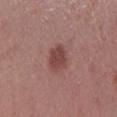This lesion was catalogued during total-body skin photography and was not selected for biopsy. The lesion's longest dimension is about 3.5 mm. This is a white-light tile. The subject is a female aged around 40. From the left lower leg. A 15 mm close-up tile from a total-body photography series done for melanoma screening.